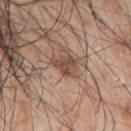notes = imaged on a skin check; not biopsied | illumination = white-light illumination | image = ~15 mm tile from a whole-body skin photo | anatomic site = the arm | subject = male, about 70 years old.